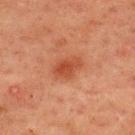Clinical impression: No biopsy was performed on this lesion — it was imaged during a full skin examination and was not determined to be concerning. Acquisition and patient details: This image is a 15 mm lesion crop taken from a total-body photograph. The lesion is on the upper back. A male subject aged approximately 60.